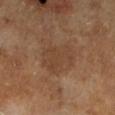Recorded during total-body skin imaging; not selected for excision or biopsy. From the left lower leg. The tile uses cross-polarized illumination. A male subject aged 63–67. An algorithmic analysis of the crop reported a footprint of about 14 mm², an eccentricity of roughly 0.6, and a shape-asymmetry score of about 0.3 (0 = symmetric). The analysis additionally found a border-irregularity rating of about 3.5/10, a within-lesion color-variation index near 2.5/10, and a peripheral color-asymmetry measure near 1. The analysis additionally found a classifier nevus-likeness of about 0/100 and lesion-presence confidence of about 100/100. Approximately 5 mm at its widest. A 15 mm close-up tile from a total-body photography series done for melanoma screening.